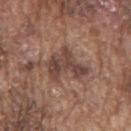Findings:
– follow-up — imaged on a skin check; not biopsied
– site — the mid back
– tile lighting — white-light illumination
– size — about 4.5 mm
– automated metrics — an average lesion color of about L≈43 a*≈18 b*≈23 (CIELAB), about 10 CIELAB-L* units darker than the surrounding skin, and a normalized lesion–skin contrast near 8; a nevus-likeness score of about 10/100 and lesion-presence confidence of about 95/100
– patient — male, roughly 75 years of age
– image — 15 mm crop, total-body photography